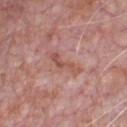Q: Is there a histopathology result?
A: total-body-photography surveillance lesion; no biopsy
Q: Lesion size?
A: ~4 mm (longest diameter)
Q: Lesion location?
A: the chest
Q: What is the imaging modality?
A: total-body-photography crop, ~15 mm field of view
Q: Patient demographics?
A: male, aged approximately 70
Q: What did automated image analysis measure?
A: a footprint of about 4 mm², an outline eccentricity of about 0.95 (0 = round, 1 = elongated), and a shape-asymmetry score of about 0.6 (0 = symmetric); border irregularity of about 7 on a 0–10 scale and peripheral color asymmetry of about 0; an automated nevus-likeness rating near 0 out of 100 and lesion-presence confidence of about 100/100